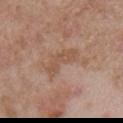workup = imaged on a skin check; not biopsied | body site = the right upper arm | acquisition = 15 mm crop, total-body photography | lesion diameter = ≈5 mm | patient = male, roughly 50 years of age | lighting = white-light illumination.